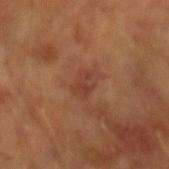Impression:
Part of a total-body skin-imaging series; this lesion was reviewed on a skin check and was not flagged for biopsy.
Image and clinical context:
This is a cross-polarized tile. A male patient, aged 63–67. Measured at roughly 3 mm in maximum diameter. An algorithmic analysis of the crop reported a color-variation rating of about 3.5/10 and a peripheral color-asymmetry measure near 1. It also reported an automated nevus-likeness rating near 0 out of 100 and lesion-presence confidence of about 100/100. A roughly 15 mm field-of-view crop from a total-body skin photograph.